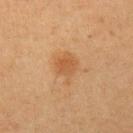Impression: The lesion was tiled from a total-body skin photograph and was not biopsied. Background: The lesion is on the left upper arm. A 15 mm close-up extracted from a 3D total-body photography capture. A female patient aged around 50. This is a cross-polarized tile.